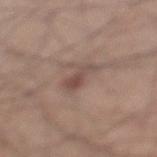The lesion was photographed on a routine skin check and not biopsied; there is no pathology result. The subject is a male roughly 30 years of age. This is a white-light tile. An algorithmic analysis of the crop reported a symmetry-axis asymmetry near 0.45. The software also gave border irregularity of about 4.5 on a 0–10 scale, a color-variation rating of about 2.5/10, and a peripheral color-asymmetry measure near 1. Approximately 3 mm at its widest. A 15 mm close-up extracted from a 3D total-body photography capture. Located on the left lower leg.